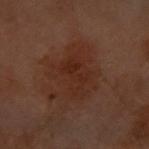The lesion was photographed on a routine skin check and not biopsied; there is no pathology result.
A female patient, aged approximately 60.
On the front of the torso.
A 15 mm crop from a total-body photograph taken for skin-cancer surveillance.
Automated image analysis of the tile measured an eccentricity of roughly 0.6 and a shape-asymmetry score of about 0.3 (0 = symmetric). And it measured a lesion color around L≈23 a*≈18 b*≈23 in CIELAB, a lesion–skin lightness drop of about 5, and a normalized lesion–skin contrast near 6. The software also gave a border-irregularity rating of about 6/10 and internal color variation of about 3 on a 0–10 scale. It also reported a nevus-likeness score of about 10/100 and lesion-presence confidence of about 100/100.